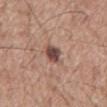Q: Was a biopsy performed?
A: catalogued during a skin exam; not biopsied
Q: Patient demographics?
A: male, aged 58 to 62
Q: What kind of image is this?
A: total-body-photography crop, ~15 mm field of view
Q: What lighting was used for the tile?
A: white-light
Q: How large is the lesion?
A: ≈3 mm
Q: What is the anatomic site?
A: the back
Q: What did automated image analysis measure?
A: an area of roughly 4.5 mm², an outline eccentricity of about 0.8 (0 = round, 1 = elongated), and a shape-asymmetry score of about 0.25 (0 = symmetric); a lesion color around L≈46 a*≈19 b*≈23 in CIELAB, a lesion–skin lightness drop of about 15, and a lesion-to-skin contrast of about 11 (normalized; higher = more distinct); a border-irregularity rating of about 2.5/10, a within-lesion color-variation index near 3.5/10, and radial color variation of about 1; a classifier nevus-likeness of about 90/100 and lesion-presence confidence of about 100/100